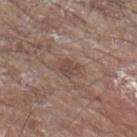Q: Is there a histopathology result?
A: total-body-photography surveillance lesion; no biopsy
Q: Lesion size?
A: about 3 mm
Q: Lesion location?
A: the left upper arm
Q: What is the imaging modality?
A: ~15 mm tile from a whole-body skin photo
Q: Automated lesion metrics?
A: a footprint of about 4.5 mm² and a symmetry-axis asymmetry near 0.2; a color-variation rating of about 2.5/10; a nevus-likeness score of about 0/100 and a detector confidence of about 100 out of 100 that the crop contains a lesion
Q: Patient demographics?
A: male, aged 78 to 82
Q: Illumination type?
A: white-light illumination